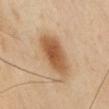{
  "biopsy_status": "not biopsied; imaged during a skin examination",
  "image": {
    "source": "total-body photography crop",
    "field_of_view_mm": 15
  },
  "patient": {
    "sex": "male",
    "age_approx": 55
  },
  "lighting": "cross-polarized",
  "automated_metrics": {
    "area_mm2_approx": 15.0,
    "eccentricity": 0.85,
    "nevus_likeness_0_100": 100,
    "lesion_detection_confidence_0_100": 100
  },
  "lesion_size": {
    "long_diameter_mm_approx": 6.0
  },
  "site": "chest"
}